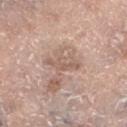Notes:
- workup — imaged on a skin check; not biopsied
- image source — 15 mm crop, total-body photography
- location — the left lower leg
- patient — female, aged 63 to 67